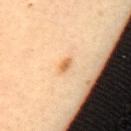biopsy_status: not biopsied; imaged during a skin examination
image:
  source: total-body photography crop
  field_of_view_mm: 15
site: mid back
patient:
  sex: female
  age_approx: 45
lighting: cross-polarized